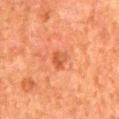Assessment:
This lesion was catalogued during total-body skin photography and was not selected for biopsy.
Acquisition and patient details:
Cropped from a whole-body photographic skin survey; the tile spans about 15 mm. The recorded lesion diameter is about 2.5 mm. A male patient in their 80s. This is a cross-polarized tile. Automated tile analysis of the lesion measured a footprint of about 3.5 mm², a shape eccentricity near 0.75, and two-axis asymmetry of about 0.3. The software also gave a border-irregularity rating of about 2.5/10, a color-variation rating of about 3/10, and a peripheral color-asymmetry measure near 1. And it measured a lesion-detection confidence of about 100/100. On the mid back.